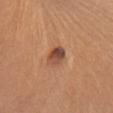{"automated_metrics": {"area_mm2_approx": 5.5, "eccentricity": 0.8, "nevus_likeness_0_100": 90}, "image": {"source": "total-body photography crop", "field_of_view_mm": 15}, "patient": {"sex": "female", "age_approx": 65}, "site": "chest"}